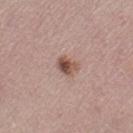Findings:
* follow-up — catalogued during a skin exam; not biopsied
* diameter — about 2.5 mm
* imaging modality — total-body-photography crop, ~15 mm field of view
* site — the left thigh
* subject — female, approximately 50 years of age
* lighting — white-light
* TBP lesion metrics — an average lesion color of about L≈51 a*≈20 b*≈25 (CIELAB) and a lesion-to-skin contrast of about 9.5 (normalized; higher = more distinct); an automated nevus-likeness rating near 90 out of 100 and a detector confidence of about 100 out of 100 that the crop contains a lesion